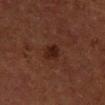Impression:
Captured during whole-body skin photography for melanoma surveillance; the lesion was not biopsied.
Context:
The lesion is located on the left forearm. A female subject, aged 48–52. About 2.5 mm across. Imaged with cross-polarized lighting. This image is a 15 mm lesion crop taken from a total-body photograph.